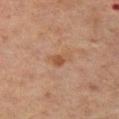- workup: total-body-photography surveillance lesion; no biopsy
- patient: male, aged 68–72
- image: ~15 mm crop, total-body skin-cancer survey
- location: the right thigh